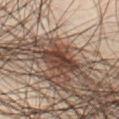The lesion was tiled from a total-body skin photograph and was not biopsied.
Approximately 5 mm at its widest.
A 15 mm crop from a total-body photograph taken for skin-cancer surveillance.
The tile uses cross-polarized illumination.
A male subject, roughly 50 years of age.
On the abdomen.